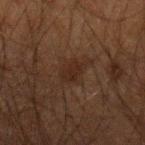The lesion was photographed on a routine skin check and not biopsied; there is no pathology result.
Imaged with cross-polarized lighting.
A male subject aged approximately 50.
A lesion tile, about 15 mm wide, cut from a 3D total-body photograph.
The lesion-visualizer software estimated a lesion area of about 5 mm² and an outline eccentricity of about 0.7 (0 = round, 1 = elongated). And it measured a mean CIELAB color near L≈19 a*≈13 b*≈19, about 5 CIELAB-L* units darker than the surrounding skin, and a normalized lesion–skin contrast near 6.
Longest diameter approximately 3 mm.
The lesion is on the left forearm.